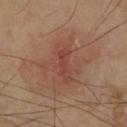Assessment:
Recorded during total-body skin imaging; not selected for excision or biopsy.
Acquisition and patient details:
The subject is a male aged approximately 65. From the arm. Captured under cross-polarized illumination. A close-up tile cropped from a whole-body skin photograph, about 15 mm across.